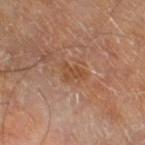workup: catalogued during a skin exam; not biopsied | acquisition: ~15 mm crop, total-body skin-cancer survey | patient: male, approximately 70 years of age | anatomic site: the right thigh.